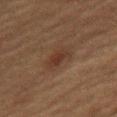Q: Was a biopsy performed?
A: total-body-photography surveillance lesion; no biopsy
Q: What is the lesion's diameter?
A: about 3 mm
Q: What did automated image analysis measure?
A: an automated nevus-likeness rating near 25 out of 100 and a lesion-detection confidence of about 100/100
Q: How was the tile lit?
A: cross-polarized
Q: Where on the body is the lesion?
A: the front of the torso
Q: What are the patient's age and sex?
A: female, approximately 70 years of age
Q: What kind of image is this?
A: ~15 mm tile from a whole-body skin photo